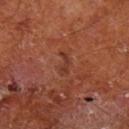Clinical impression: The lesion was tiled from a total-body skin photograph and was not biopsied. Acquisition and patient details: Approximately 3 mm at its widest. A 15 mm close-up extracted from a 3D total-body photography capture. A male patient in their mid-60s. Automated image analysis of the tile measured an average lesion color of about L≈34 a*≈24 b*≈28 (CIELAB), a lesion–skin lightness drop of about 7, and a normalized border contrast of about 6. And it measured a border-irregularity index near 7.5/10 and a peripheral color-asymmetry measure near 0. And it measured an automated nevus-likeness rating near 0 out of 100. The tile uses cross-polarized illumination. Located on the leg.